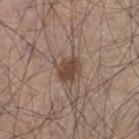Recorded during total-body skin imaging; not selected for excision or biopsy. A roughly 15 mm field-of-view crop from a total-body skin photograph. This is a white-light tile. A male subject, about 45 years old. About 3.5 mm across. From the leg.